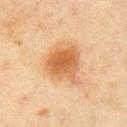biopsy status — catalogued during a skin exam; not biopsied
site — the right upper arm
subject — male, roughly 45 years of age
acquisition — ~15 mm tile from a whole-body skin photo